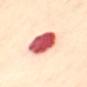The lesion was tiled from a total-body skin photograph and was not biopsied. An algorithmic analysis of the crop reported a lesion area of about 15 mm² and a shape eccentricity near 0.55. And it measured an average lesion color of about L≈62 a*≈39 b*≈29 (CIELAB) and a normalized lesion–skin contrast near 15. Located on the mid back. The subject is a female roughly 55 years of age. Approximately 5 mm at its widest. This is a cross-polarized tile. A close-up tile cropped from a whole-body skin photograph, about 15 mm across.